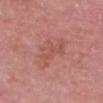Assessment:
The lesion was tiled from a total-body skin photograph and was not biopsied.
Acquisition and patient details:
The recorded lesion diameter is about 5 mm. A male patient in their mid-70s. Located on the head or neck. Imaged with white-light lighting. Cropped from a total-body skin-imaging series; the visible field is about 15 mm. Automated image analysis of the tile measured an area of roughly 9 mm², an eccentricity of roughly 0.9, and a shape-asymmetry score of about 0.45 (0 = symmetric). It also reported a border-irregularity index near 6/10, internal color variation of about 2.5 on a 0–10 scale, and a peripheral color-asymmetry measure near 1.